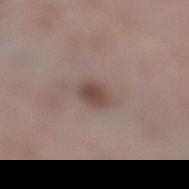Clinical impression:
Captured during whole-body skin photography for melanoma surveillance; the lesion was not biopsied.
Image and clinical context:
The tile uses white-light illumination. A lesion tile, about 15 mm wide, cut from a 3D total-body photograph. The recorded lesion diameter is about 3 mm. The lesion is located on the left lower leg. A male patient, aged 38–42.